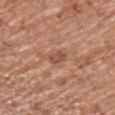<lesion>
<biopsy_status>not biopsied; imaged during a skin examination</biopsy_status>
<automated_metrics>
  <area_mm2_approx>3.0</area_mm2_approx>
  <shape_asymmetry>0.2</shape_asymmetry>
</automated_metrics>
<lighting>white-light</lighting>
<site>left upper arm</site>
<image>
  <source>total-body photography crop</source>
  <field_of_view_mm>15</field_of_view_mm>
</image>
<patient>
  <sex>male</sex>
  <age_approx>65</age_approx>
</patient>
</lesion>